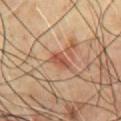Q: Was a biopsy performed?
A: no biopsy performed (imaged during a skin exam)
Q: Automated lesion metrics?
A: a footprint of about 3.5 mm², an outline eccentricity of about 0.75 (0 = round, 1 = elongated), and a shape-asymmetry score of about 0.3 (0 = symmetric); a mean CIELAB color near L≈50 a*≈25 b*≈31, roughly 9 lightness units darker than nearby skin, and a normalized lesion–skin contrast near 7; a within-lesion color-variation index near 2/10; a lesion-detection confidence of about 100/100
Q: What kind of image is this?
A: ~15 mm crop, total-body skin-cancer survey
Q: What lighting was used for the tile?
A: cross-polarized
Q: Who is the patient?
A: male, aged 68–72
Q: Lesion size?
A: ≈2.5 mm
Q: Lesion location?
A: the chest